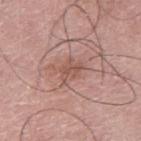The lesion was photographed on a routine skin check and not biopsied; there is no pathology result. Approximately 3 mm at its widest. From the left thigh. A male subject, approximately 75 years of age. A close-up tile cropped from a whole-body skin photograph, about 15 mm across. Imaged with white-light lighting. The lesion-visualizer software estimated a lesion area of about 5 mm², an outline eccentricity of about 0.7 (0 = round, 1 = elongated), and a shape-asymmetry score of about 0.45 (0 = symmetric). The analysis additionally found a border-irregularity index near 5/10, a within-lesion color-variation index near 2.5/10, and radial color variation of about 1.